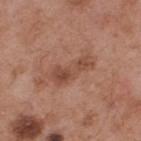– follow-up: total-body-photography surveillance lesion; no biopsy
– lesion diameter: about 5 mm
– automated lesion analysis: a lesion color around L≈48 a*≈22 b*≈29 in CIELAB, roughly 9 lightness units darker than nearby skin, and a normalized lesion–skin contrast near 6.5; a border-irregularity rating of about 5/10, internal color variation of about 4.5 on a 0–10 scale, and a peripheral color-asymmetry measure near 1.5
– subject: male, aged around 55
– imaging modality: ~15 mm tile from a whole-body skin photo
– site: the upper back